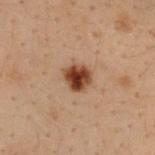| feature | finding |
|---|---|
| lesion diameter | about 3 mm |
| tile lighting | cross-polarized illumination |
| TBP lesion metrics | a footprint of about 7 mm², a shape eccentricity near 0.15, and two-axis asymmetry of about 0.2 |
| subject | male, aged 28–32 |
| site | the upper back |
| image source | 15 mm crop, total-body photography |